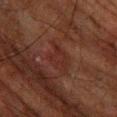notes = no biopsy performed (imaged during a skin exam)
location = the left forearm
illumination = cross-polarized
size = about 4 mm
subject = male, aged 63 to 67
imaging modality = total-body-photography crop, ~15 mm field of view
image-analysis metrics = an average lesion color of about L≈22 a*≈19 b*≈20 (CIELAB) and roughly 4 lightness units darker than nearby skin; border irregularity of about 4.5 on a 0–10 scale and a color-variation rating of about 2/10